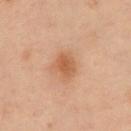The lesion's longest dimension is about 3 mm. The tile uses cross-polarized illumination. On the chest. Automated image analysis of the tile measured an area of roughly 6.5 mm², an eccentricity of roughly 0.6, and a symmetry-axis asymmetry near 0.25. The software also gave a mean CIELAB color near L≈46 a*≈19 b*≈30, a lesion–skin lightness drop of about 8, and a lesion-to-skin contrast of about 7 (normalized; higher = more distinct). The software also gave an automated nevus-likeness rating near 70 out of 100. A 15 mm close-up tile from a total-body photography series done for melanoma screening. The patient is a male aged 53 to 57.